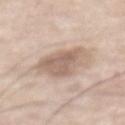Part of a total-body skin-imaging series; this lesion was reviewed on a skin check and was not flagged for biopsy.